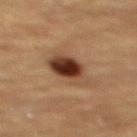– notes — total-body-photography surveillance lesion; no biopsy
– diameter — ~5 mm (longest diameter)
– subject — female, roughly 70 years of age
– illumination — cross-polarized illumination
– location — the back
– image — 15 mm crop, total-body photography